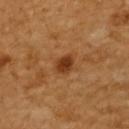Case summary:
- image — ~15 mm crop, total-body skin-cancer survey
- body site — the back
- automated metrics — a footprint of about 5 mm², an eccentricity of roughly 0.6, and a shape-asymmetry score of about 0.15 (0 = symmetric); a classifier nevus-likeness of about 90/100 and lesion-presence confidence of about 100/100
- patient — female, about 55 years old
- lesion diameter — about 3 mm